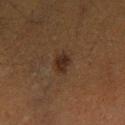biopsy_status: not biopsied; imaged during a skin examination
lighting: cross-polarized
patient:
  sex: male
  age_approx: 60
lesion_size:
  long_diameter_mm_approx: 2.5
site: leg
image:
  source: total-body photography crop
  field_of_view_mm: 15